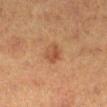Assessment:
The lesion was photographed on a routine skin check and not biopsied; there is no pathology result.
Acquisition and patient details:
The lesion is on the right lower leg. Cropped from a total-body skin-imaging series; the visible field is about 15 mm. A female subject, roughly 40 years of age. The lesion's longest dimension is about 2.5 mm. Imaged with cross-polarized lighting.